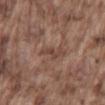Assessment: Recorded during total-body skin imaging; not selected for excision or biopsy. Background: The lesion-visualizer software estimated a shape-asymmetry score of about 0.4 (0 = symmetric). It also reported a color-variation rating of about 0/10 and a peripheral color-asymmetry measure near 0. The software also gave an automated nevus-likeness rating near 0 out of 100 and lesion-presence confidence of about 100/100. A male patient, in their mid- to late 70s. Captured under white-light illumination. Cropped from a total-body skin-imaging series; the visible field is about 15 mm. Located on the mid back. About 3 mm across.